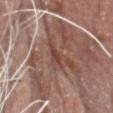The lesion was photographed on a routine skin check and not biopsied; there is no pathology result.
A 15 mm close-up tile from a total-body photography series done for melanoma screening.
About 2.5 mm across.
The lesion is on the head or neck.
Captured under white-light illumination.
A male subject in their 70s.